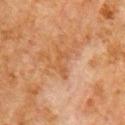diameter: about 3.5 mm | site: the right upper arm | subject: male, aged around 80 | lighting: cross-polarized | imaging modality: 15 mm crop, total-body photography.